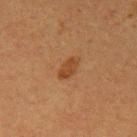Case summary:
* follow-up · total-body-photography surveillance lesion; no biopsy
* patient · female, approximately 55 years of age
* site · the left upper arm
* image · ~15 mm tile from a whole-body skin photo